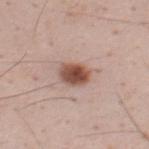diameter: ≈3.5 mm | tile lighting: white-light illumination | image source: ~15 mm crop, total-body skin-cancer survey | patient: male, about 35 years old | body site: the upper back.